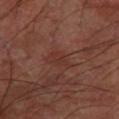Notes:
- workup: total-body-photography surveillance lesion; no biopsy
- image: ~15 mm tile from a whole-body skin photo
- subject: male, aged 63 to 67
- body site: the left forearm